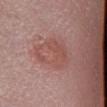| key | value |
|---|---|
| biopsy status | no biopsy performed (imaged during a skin exam) |
| automated metrics | a border-irregularity rating of about 3/10 and a color-variation rating of about 4/10; a nevus-likeness score of about 15/100 and lesion-presence confidence of about 100/100 |
| image | ~15 mm crop, total-body skin-cancer survey |
| lighting | white-light |
| diameter | ~6 mm (longest diameter) |
| patient | male, about 55 years old |
| site | the leg |